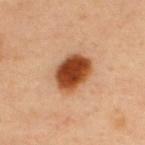Part of a total-body skin-imaging series; this lesion was reviewed on a skin check and was not flagged for biopsy. On the upper back. A 15 mm close-up tile from a total-body photography series done for melanoma screening. A male patient, aged around 45.